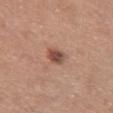| field | value |
|---|---|
| follow-up | no biopsy performed (imaged during a skin exam) |
| image | ~15 mm crop, total-body skin-cancer survey |
| patient | female, aged around 60 |
| site | the chest |